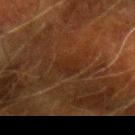Recorded during total-body skin imaging; not selected for excision or biopsy. The lesion is on the left forearm. An algorithmic analysis of the crop reported a lesion area of about 4.5 mm², an outline eccentricity of about 0.7 (0 = round, 1 = elongated), and a shape-asymmetry score of about 0.25 (0 = symmetric). It also reported a lesion–skin lightness drop of about 4. The software also gave a border-irregularity rating of about 2.5/10, a color-variation rating of about 2/10, and a peripheral color-asymmetry measure near 1. It also reported a classifier nevus-likeness of about 0/100 and lesion-presence confidence of about 60/100. A male patient, approximately 65 years of age. A 15 mm crop from a total-body photograph taken for skin-cancer surveillance. About 3 mm across.